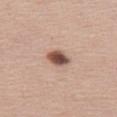Imaged during a routine full-body skin examination; the lesion was not biopsied and no histopathology is available. This image is a 15 mm lesion crop taken from a total-body photograph. Located on the left thigh. The lesion-visualizer software estimated a border-irregularity index near 1.5/10 and internal color variation of about 6 on a 0–10 scale. And it measured a classifier nevus-likeness of about 100/100. The subject is a female aged approximately 40. Captured under white-light illumination. Approximately 3 mm at its widest.